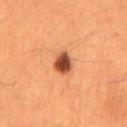biopsy status — total-body-photography surveillance lesion; no biopsy | acquisition — 15 mm crop, total-body photography | subject — male, aged 48 to 52 | anatomic site — the mid back | size — ≈3 mm | illumination — cross-polarized illumination.